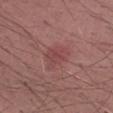Assessment:
The lesion was tiled from a total-body skin photograph and was not biopsied.
Context:
Imaged with white-light lighting. A male subject, roughly 50 years of age. The lesion's longest dimension is about 4 mm. The lesion-visualizer software estimated a lesion area of about 7.5 mm², an eccentricity of roughly 0.75, and a shape-asymmetry score of about 0.35 (0 = symmetric). And it measured a mean CIELAB color near L≈45 a*≈26 b*≈21 and a normalized border contrast of about 5. And it measured border irregularity of about 3.5 on a 0–10 scale, internal color variation of about 2 on a 0–10 scale, and radial color variation of about 0.5. The software also gave an automated nevus-likeness rating near 5 out of 100 and lesion-presence confidence of about 100/100. A roughly 15 mm field-of-view crop from a total-body skin photograph. The lesion is on the right upper arm.